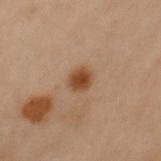Part of a total-body skin-imaging series; this lesion was reviewed on a skin check and was not flagged for biopsy. Cropped from a whole-body photographic skin survey; the tile spans about 15 mm. A female subject aged 58 to 62. On the right arm. The lesion-visualizer software estimated an outline eccentricity of about 0.6 (0 = round, 1 = elongated) and a shape-asymmetry score of about 0.25 (0 = symmetric). The analysis additionally found a nevus-likeness score of about 100/100 and a lesion-detection confidence of about 100/100. Imaged with cross-polarized lighting. Approximately 2.5 mm at its widest.